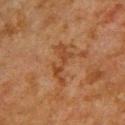<lesion>
  <site>back</site>
  <automated_metrics>
    <area_mm2_approx>7.0</area_mm2_approx>
    <shape_asymmetry>0.6</shape_asymmetry>
    <cielab_L>36</cielab_L>
    <cielab_a>20</cielab_a>
    <cielab_b>31</cielab_b>
    <vs_skin_darker_L>6.0</vs_skin_darker_L>
    <vs_skin_contrast_norm>6.0</vs_skin_contrast_norm>
    <nevus_likeness_0_100>0</nevus_likeness_0_100>
    <lesion_detection_confidence_0_100>100</lesion_detection_confidence_0_100>
  </automated_metrics>
  <lesion_size>
    <long_diameter_mm_approx>5.0</long_diameter_mm_approx>
  </lesion_size>
  <image>
    <source>total-body photography crop</source>
    <field_of_view_mm>15</field_of_view_mm>
  </image>
  <lighting>cross-polarized</lighting>
  <patient>
    <sex>male</sex>
    <age_approx>65</age_approx>
  </patient>
</lesion>